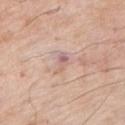Image and clinical context: About 3 mm across. A male patient aged around 70. A region of skin cropped from a whole-body photographic capture, roughly 15 mm wide. The lesion is on the left upper arm. Captured under white-light illumination.